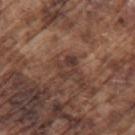Impression:
Recorded during total-body skin imaging; not selected for excision or biopsy.
Acquisition and patient details:
A male patient in their mid-70s. Approximately 3 mm at its widest. The lesion is located on the left upper arm. This is a white-light tile. A 15 mm crop from a total-body photograph taken for skin-cancer surveillance.